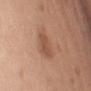About 3.5 mm across. Automated image analysis of the tile measured a lesion color around L≈52 a*≈22 b*≈31 in CIELAB, about 8 CIELAB-L* units darker than the surrounding skin, and a lesion-to-skin contrast of about 6 (normalized; higher = more distinct). It also reported border irregularity of about 2 on a 0–10 scale. And it measured an automated nevus-likeness rating near 20 out of 100. On the chest. A female patient, about 55 years old. A 15 mm close-up extracted from a 3D total-body photography capture. This is a white-light tile.